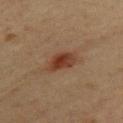Q: Is there a histopathology result?
A: no biopsy performed (imaged during a skin exam)